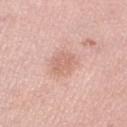location = the left lower leg | size = ~3 mm (longest diameter) | image source = total-body-photography crop, ~15 mm field of view | patient = female, aged around 65.